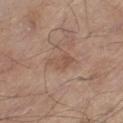| feature | finding |
|---|---|
| biopsy status | catalogued during a skin exam; not biopsied |
| lighting | white-light |
| patient | male, about 80 years old |
| body site | the left thigh |
| image | ~15 mm crop, total-body skin-cancer survey |